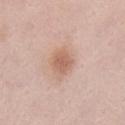Part of a total-body skin-imaging series; this lesion was reviewed on a skin check and was not flagged for biopsy.
On the chest.
A female patient, approximately 40 years of age.
Longest diameter approximately 2.5 mm.
A 15 mm close-up extracted from a 3D total-body photography capture.